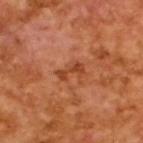A 15 mm close-up extracted from a 3D total-body photography capture. The patient is a male aged approximately 65.